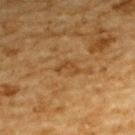Recorded during total-body skin imaging; not selected for excision or biopsy. Cropped from a total-body skin-imaging series; the visible field is about 15 mm. Automated image analysis of the tile measured an area of roughly 2.5 mm² and an outline eccentricity of about 0.85 (0 = round, 1 = elongated). And it measured a border-irregularity index near 7/10. And it measured a nevus-likeness score of about 0/100. From the upper back. Measured at roughly 3 mm in maximum diameter. A male patient aged 83 or over. This is a cross-polarized tile.